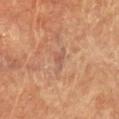follow-up: no biopsy performed (imaged during a skin exam)
TBP lesion metrics: a footprint of about 2.5 mm², an outline eccentricity of about 0.85 (0 = round, 1 = elongated), and two-axis asymmetry of about 0.5; a lesion color around L≈50 a*≈21 b*≈27 in CIELAB, a lesion–skin lightness drop of about 6, and a normalized border contrast of about 5; border irregularity of about 4.5 on a 0–10 scale, a within-lesion color-variation index near 1.5/10, and a peripheral color-asymmetry measure near 0.5; a nevus-likeness score of about 0/100
image source: total-body-photography crop, ~15 mm field of view
anatomic site: the chest
size: ≈2.5 mm
subject: female, aged 78 to 82